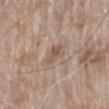{
  "biopsy_status": "not biopsied; imaged during a skin examination",
  "site": "mid back",
  "patient": {
    "sex": "male",
    "age_approx": 80
  },
  "automated_metrics": {
    "area_mm2_approx": 3.5,
    "shape_asymmetry": 0.35
  },
  "image": {
    "source": "total-body photography crop",
    "field_of_view_mm": 15
  },
  "lesion_size": {
    "long_diameter_mm_approx": 2.5
  },
  "lighting": "white-light"
}